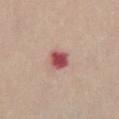biopsy status: catalogued during a skin exam; not biopsied.